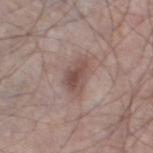This lesion was catalogued during total-body skin photography and was not selected for biopsy. The patient is a male in their mid-60s. The recorded lesion diameter is about 4 mm. Cropped from a total-body skin-imaging series; the visible field is about 15 mm. From the left thigh. This is a white-light tile. Automated image analysis of the tile measured a lesion area of about 8.5 mm², an outline eccentricity of about 0.8 (0 = round, 1 = elongated), and a symmetry-axis asymmetry near 0.35. The analysis additionally found a classifier nevus-likeness of about 25/100 and a detector confidence of about 100 out of 100 that the crop contains a lesion.